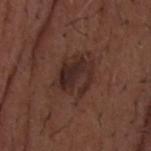biopsy status: total-body-photography surveillance lesion; no biopsy
body site: the head or neck
acquisition: ~15 mm tile from a whole-body skin photo
subject: male, aged 48 to 52
illumination: white-light